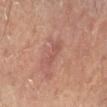Impression: No biopsy was performed on this lesion — it was imaged during a full skin examination and was not determined to be concerning. Clinical summary: Located on the left lower leg. This is a cross-polarized tile. A close-up tile cropped from a whole-body skin photograph, about 15 mm across. A male patient roughly 65 years of age.